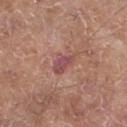{"biopsy_status": "not biopsied; imaged during a skin examination", "image": {"source": "total-body photography crop", "field_of_view_mm": 15}, "patient": {"sex": "male", "age_approx": 65}, "automated_metrics": {"area_mm2_approx": 4.5, "eccentricity": 0.8, "shape_asymmetry": 0.3, "cielab_L": 49, "cielab_a": 25, "cielab_b": 22, "vs_skin_darker_L": 9.0, "vs_skin_contrast_norm": 7.0}, "site": "leg"}